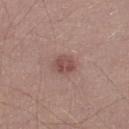notes = total-body-photography surveillance lesion; no biopsy
diameter = ~2.5 mm (longest diameter)
anatomic site = the left lower leg
subject = male, in their 40s
tile lighting = white-light
imaging modality = ~15 mm tile from a whole-body skin photo